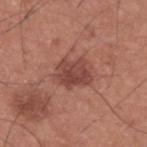Impression: Part of a total-body skin-imaging series; this lesion was reviewed on a skin check and was not flagged for biopsy. Image and clinical context: A male subject approximately 35 years of age. The lesion's longest dimension is about 4 mm. The lesion-visualizer software estimated a lesion area of about 10 mm², a shape eccentricity near 0.6, and two-axis asymmetry of about 0.25. The software also gave a lesion color around L≈45 a*≈25 b*≈25 in CIELAB. And it measured a border-irregularity index near 3/10, a color-variation rating of about 3.5/10, and peripheral color asymmetry of about 1.5. And it measured an automated nevus-likeness rating near 35 out of 100. The lesion is located on the upper back. A roughly 15 mm field-of-view crop from a total-body skin photograph.